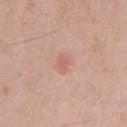workup: imaged on a skin check; not biopsied
patient: male, aged around 70
lesion diameter: ~2.5 mm (longest diameter)
site: the chest
automated metrics: a lesion area of about 2.5 mm² and two-axis asymmetry of about 0.25; an average lesion color of about L≈61 a*≈23 b*≈29 (CIELAB) and roughly 7 lightness units darker than nearby skin; a border-irregularity index near 3/10, internal color variation of about 0 on a 0–10 scale, and peripheral color asymmetry of about 0; an automated nevus-likeness rating near 5 out of 100 and a detector confidence of about 100 out of 100 that the crop contains a lesion
image: total-body-photography crop, ~15 mm field of view